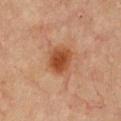The lesion was tiled from a total-body skin photograph and was not biopsied.
The recorded lesion diameter is about 3.5 mm.
A 15 mm crop from a total-body photograph taken for skin-cancer surveillance.
The subject is a female roughly 65 years of age.
The lesion is located on the chest.
The total-body-photography lesion software estimated an average lesion color of about L≈42 a*≈23 b*≈33 (CIELAB), about 10 CIELAB-L* units darker than the surrounding skin, and a normalized lesion–skin contrast near 9.